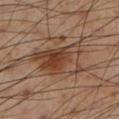This lesion was catalogued during total-body skin photography and was not selected for biopsy. A male patient, aged 53 to 57. From the leg. A 15 mm close-up tile from a total-body photography series done for melanoma screening.